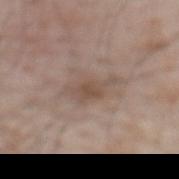Notes:
– follow-up · no biopsy performed (imaged during a skin exam)
– illumination · white-light
– body site · the mid back
– diameter · ≈4.5 mm
– subject · male, aged 63 to 67
– image · 15 mm crop, total-body photography